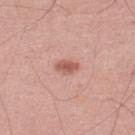Clinical impression:
Recorded during total-body skin imaging; not selected for excision or biopsy.
Acquisition and patient details:
The subject is a male approximately 50 years of age. Located on the left lower leg. Captured under white-light illumination. The lesion's longest dimension is about 2.5 mm. A 15 mm close-up tile from a total-body photography series done for melanoma screening.